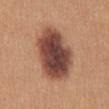Captured during whole-body skin photography for melanoma surveillance; the lesion was not biopsied.
A region of skin cropped from a whole-body photographic capture, roughly 15 mm wide.
A female patient, about 40 years old.
Imaged with white-light lighting.
Located on the mid back.